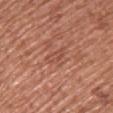Assessment: The lesion was photographed on a routine skin check and not biopsied; there is no pathology result. Background: Cropped from a total-body skin-imaging series; the visible field is about 15 mm. Captured under white-light illumination. On the upper back. A female subject, roughly 60 years of age.